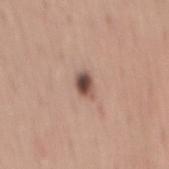The lesion was tiled from a total-body skin photograph and was not biopsied. On the lower back. A male subject, aged 43–47. A lesion tile, about 15 mm wide, cut from a 3D total-body photograph. The lesion-visualizer software estimated a lesion color around L≈49 a*≈19 b*≈24 in CIELAB, a lesion–skin lightness drop of about 17, and a normalized border contrast of about 11.5. It also reported a border-irregularity index near 2/10.